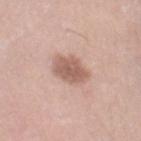<lesion>
<lesion_size>
  <long_diameter_mm_approx>4.0</long_diameter_mm_approx>
</lesion_size>
<automated_metrics>
  <eccentricity>0.55</eccentricity>
  <shape_asymmetry>0.15</shape_asymmetry>
  <cielab_L>59</cielab_L>
  <cielab_a>19</cielab_a>
  <cielab_b>26</cielab_b>
  <vs_skin_darker_L>12.0</vs_skin_darker_L>
  <vs_skin_contrast_norm>7.5</vs_skin_contrast_norm>
  <border_irregularity_0_10>2.0</border_irregularity_0_10>
  <color_variation_0_10>3.0</color_variation_0_10>
  <peripheral_color_asymmetry>1.0</peripheral_color_asymmetry>
  <nevus_likeness_0_100>40</nevus_likeness_0_100>
  <lesion_detection_confidence_0_100>100</lesion_detection_confidence_0_100>
</automated_metrics>
<image>
  <source>total-body photography crop</source>
  <field_of_view_mm>15</field_of_view_mm>
</image>
<site>right thigh</site>
<patient>
  <sex>female</sex>
  <age_approx>40</age_approx>
</patient>
</lesion>